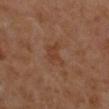* tile lighting — cross-polarized
* anatomic site — the left forearm
* subject — female, roughly 50 years of age
* imaging modality — ~15 mm tile from a whole-body skin photo
* lesion diameter — ~3 mm (longest diameter)